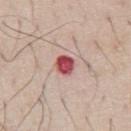Context:
On the chest. A 15 mm crop from a total-body photograph taken for skin-cancer surveillance. A male patient aged around 70.